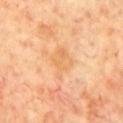This lesion was catalogued during total-body skin photography and was not selected for biopsy. The tile uses cross-polarized illumination. A male subject, aged approximately 70. A lesion tile, about 15 mm wide, cut from a 3D total-body photograph. The lesion is located on the front of the torso. Approximately 4 mm at its widest.